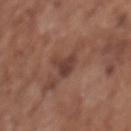No biopsy was performed on this lesion — it was imaged during a full skin examination and was not determined to be concerning. Longest diameter approximately 3.5 mm. Imaged with white-light lighting. From the upper back. A 15 mm close-up extracted from a 3D total-body photography capture. A female patient aged 73 to 77.